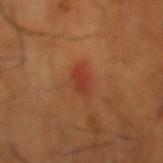Impression: The lesion was photographed on a routine skin check and not biopsied; there is no pathology result. Background: Located on the left lower leg. A male patient aged 63–67. A 15 mm close-up tile from a total-body photography series done for melanoma screening.